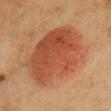Assessment:
Captured during whole-body skin photography for melanoma surveillance; the lesion was not biopsied.
Image and clinical context:
A 15 mm close-up extracted from a 3D total-body photography capture. From the chest. Automated image analysis of the tile measured a lesion area of about 44 mm², a shape eccentricity near 0.35, and a shape-asymmetry score of about 0.15 (0 = symmetric). And it measured a border-irregularity index near 2/10, a color-variation rating of about 4.5/10, and peripheral color asymmetry of about 1.5. Imaged with cross-polarized lighting. A female subject, in their 50s.